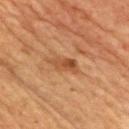<lesion>
  <biopsy_status>not biopsied; imaged during a skin examination</biopsy_status>
  <image>
    <source>total-body photography crop</source>
    <field_of_view_mm>15</field_of_view_mm>
  </image>
  <lighting>cross-polarized</lighting>
  <lesion_size>
    <long_diameter_mm_approx>4.0</long_diameter_mm_approx>
  </lesion_size>
  <patient>
    <sex>male</sex>
    <age_approx>65</age_approx>
  </patient>
  <site>chest</site>
</lesion>